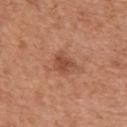Recorded during total-body skin imaging; not selected for excision or biopsy. This image is a 15 mm lesion crop taken from a total-body photograph. Imaged with white-light lighting. On the front of the torso. Longest diameter approximately 3 mm. The patient is a male about 65 years old.